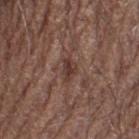The lesion was photographed on a routine skin check and not biopsied; there is no pathology result. Approximately 2.5 mm at its widest. This is a white-light tile. A male patient, in their 70s. The lesion is located on the left thigh. A 15 mm close-up extracted from a 3D total-body photography capture.